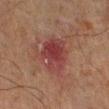Part of a total-body skin-imaging series; this lesion was reviewed on a skin check and was not flagged for biopsy.
The lesion is located on the left lower leg.
The lesion-visualizer software estimated an area of roughly 14 mm², an outline eccentricity of about 0.6 (0 = round, 1 = elongated), and a symmetry-axis asymmetry near 0.25. It also reported a lesion–skin lightness drop of about 7 and a lesion-to-skin contrast of about 8 (normalized; higher = more distinct). The software also gave border irregularity of about 3 on a 0–10 scale and a within-lesion color-variation index near 4/10.
A close-up tile cropped from a whole-body skin photograph, about 15 mm across.
The subject is a male aged 63–67.
Imaged with cross-polarized lighting.